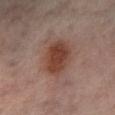biopsy_status: not biopsied; imaged during a skin examination
site: left lower leg
patient:
  sex: male
  age_approx: 40
image:
  source: total-body photography crop
  field_of_view_mm: 15